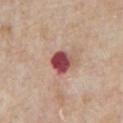notes = catalogued during a skin exam; not biopsied
patient = male, in their mid-60s
body site = the chest
imaging modality = 15 mm crop, total-body photography